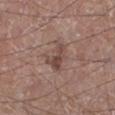notes = total-body-photography surveillance lesion; no biopsy.